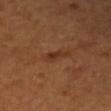Recorded during total-body skin imaging; not selected for excision or biopsy. Located on the right forearm. This image is a 15 mm lesion crop taken from a total-body photograph. About 2.5 mm across. The patient is a female aged 53 to 57. Captured under cross-polarized illumination. An algorithmic analysis of the crop reported a footprint of about 3 mm², a shape eccentricity near 0.9, and a symmetry-axis asymmetry near 0.45. The analysis additionally found a lesion–skin lightness drop of about 7 and a normalized border contrast of about 6.5. It also reported a lesion-detection confidence of about 100/100.